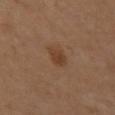Captured during whole-body skin photography for melanoma surveillance; the lesion was not biopsied.
The subject is a female aged 53 to 57.
On the leg.
A close-up tile cropped from a whole-body skin photograph, about 15 mm across.